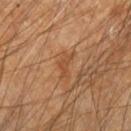Findings:
- biopsy status · imaged on a skin check; not biopsied
- illumination · cross-polarized illumination
- lesion diameter · ≈2.5 mm
- image source · 15 mm crop, total-body photography
- subject · male, approximately 60 years of age
- automated metrics · border irregularity of about 6 on a 0–10 scale and a within-lesion color-variation index near 0/10
- anatomic site · the left forearm